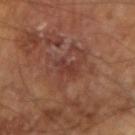The lesion was tiled from a total-body skin photograph and was not biopsied. A lesion tile, about 15 mm wide, cut from a 3D total-body photograph. The tile uses cross-polarized illumination. From the left arm. The subject is a male aged 63–67. The lesion's longest dimension is about 3 mm. The lesion-visualizer software estimated a lesion area of about 4 mm², an outline eccentricity of about 0.75 (0 = round, 1 = elongated), and two-axis asymmetry of about 0.35. The analysis additionally found a lesion color around L≈35 a*≈23 b*≈24 in CIELAB. It also reported a border-irregularity rating of about 4/10 and a color-variation rating of about 1.5/10. The software also gave an automated nevus-likeness rating near 0 out of 100.